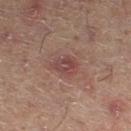Q: Was a biopsy performed?
A: no biopsy performed (imaged during a skin exam)
Q: How was this image acquired?
A: total-body-photography crop, ~15 mm field of view
Q: Patient demographics?
A: male, approximately 50 years of age
Q: Automated lesion metrics?
A: an average lesion color of about L≈38 a*≈20 b*≈19 (CIELAB), a lesion–skin lightness drop of about 7, and a lesion-to-skin contrast of about 6.5 (normalized; higher = more distinct)
Q: How was the tile lit?
A: cross-polarized illumination
Q: How large is the lesion?
A: about 3 mm
Q: Where on the body is the lesion?
A: the leg